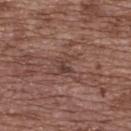Q: Was a biopsy performed?
A: imaged on a skin check; not biopsied
Q: What is the imaging modality?
A: 15 mm crop, total-body photography
Q: How was the tile lit?
A: white-light illumination
Q: Lesion location?
A: the upper back
Q: Who is the patient?
A: female, aged 73 to 77
Q: Lesion size?
A: ≈3 mm